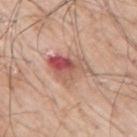Captured during whole-body skin photography for melanoma surveillance; the lesion was not biopsied.
Cropped from a total-body skin-imaging series; the visible field is about 15 mm.
The lesion is located on the right upper arm.
A male patient, approximately 70 years of age.